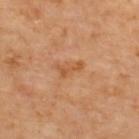This lesion was catalogued during total-body skin photography and was not selected for biopsy. Automated tile analysis of the lesion measured a border-irregularity rating of about 6.5/10, a color-variation rating of about 0/10, and peripheral color asymmetry of about 0. This is a cross-polarized tile. A region of skin cropped from a whole-body photographic capture, roughly 15 mm wide. On the back. Longest diameter approximately 3 mm.